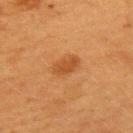{"biopsy_status": "not biopsied; imaged during a skin examination", "lesion_size": {"long_diameter_mm_approx": 3.5}, "site": "upper back", "patient": {"sex": "female", "age_approx": 40}, "image": {"source": "total-body photography crop", "field_of_view_mm": 15}}